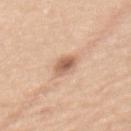workup=total-body-photography surveillance lesion; no biopsy
site=the mid back
subject=female, in their mid-60s
lesion size=≈3.5 mm
image=~15 mm tile from a whole-body skin photo